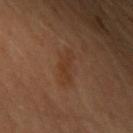biopsy status — total-body-photography surveillance lesion; no biopsy | image source — total-body-photography crop, ~15 mm field of view | body site — the left upper arm | illumination — cross-polarized | subject — male, aged 38–42.